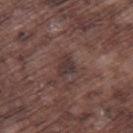Captured during whole-body skin photography for melanoma surveillance; the lesion was not biopsied.
Imaged with white-light lighting.
A 15 mm crop from a total-body photograph taken for skin-cancer surveillance.
The subject is a male in their mid- to late 70s.
Located on the right thigh.